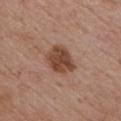Approximately 3.5 mm at its widest. The tile uses white-light illumination. Located on the chest. A 15 mm crop from a total-body photograph taken for skin-cancer surveillance. The subject is a male roughly 75 years of age. The total-body-photography lesion software estimated roughly 13 lightness units darker than nearby skin. It also reported border irregularity of about 2 on a 0–10 scale and a within-lesion color-variation index near 3.5/10.